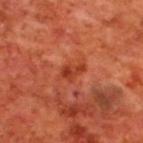<case>
<image>
  <source>total-body photography crop</source>
  <field_of_view_mm>15</field_of_view_mm>
</image>
<patient>
  <sex>male</sex>
  <age_approx>70</age_approx>
</patient>
<site>upper back</site>
</case>